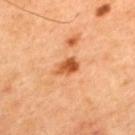patient: male, aged 43 to 47
image-analysis metrics: a mean CIELAB color near L≈55 a*≈29 b*≈42, about 13 CIELAB-L* units darker than the surrounding skin, and a normalized border contrast of about 9; an automated nevus-likeness rating near 85 out of 100
diameter: about 3.5 mm
body site: the back
imaging modality: 15 mm crop, total-body photography